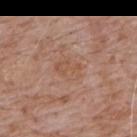No biopsy was performed on this lesion — it was imaged during a full skin examination and was not determined to be concerning. The patient is a male aged approximately 60. Measured at roughly 3 mm in maximum diameter. Cropped from a total-body skin-imaging series; the visible field is about 15 mm. From the mid back. The tile uses white-light illumination.